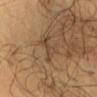Assessment:
Imaged during a routine full-body skin examination; the lesion was not biopsied and no histopathology is available.
Clinical summary:
From the chest. A close-up tile cropped from a whole-body skin photograph, about 15 mm across. The patient is a male aged 53 to 57.